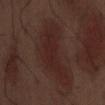Assessment: Captured during whole-body skin photography for melanoma surveillance; the lesion was not biopsied. Acquisition and patient details: The lesion-visualizer software estimated a lesion area of about 23 mm², an eccentricity of roughly 0.85, and a symmetry-axis asymmetry near 0.55. It also reported a border-irregularity index near 6.5/10, a color-variation rating of about 2.5/10, and peripheral color asymmetry of about 1. A male patient aged 68–72. Cropped from a total-body skin-imaging series; the visible field is about 15 mm. The lesion is located on the abdomen. Captured under white-light illumination. Measured at roughly 7.5 mm in maximum diameter.